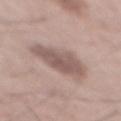{
  "site": "mid back",
  "lighting": "white-light",
  "lesion_size": {
    "long_diameter_mm_approx": 6.0
  },
  "image": {
    "source": "total-body photography crop",
    "field_of_view_mm": 15
  },
  "patient": {
    "sex": "male",
    "age_approx": 55
  },
  "automated_metrics": {
    "area_mm2_approx": 16.0,
    "eccentricity": 0.8,
    "nevus_likeness_0_100": 20,
    "lesion_detection_confidence_0_100": 100
  }
}